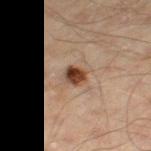Findings:
- follow-up · no biopsy performed (imaged during a skin exam)
- illumination · cross-polarized illumination
- image source · 15 mm crop, total-body photography
- automated metrics · a border-irregularity rating of about 1.5/10, a within-lesion color-variation index near 6.5/10, and radial color variation of about 2.5; a nevus-likeness score of about 100/100 and a detector confidence of about 100 out of 100 that the crop contains a lesion
- diameter · ≈2.5 mm
- patient · male, about 45 years old
- anatomic site · the left thigh